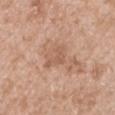Clinical impression: Imaged during a routine full-body skin examination; the lesion was not biopsied and no histopathology is available. Clinical summary: From the right upper arm. Cropped from a total-body skin-imaging series; the visible field is about 15 mm. Measured at roughly 3 mm in maximum diameter. An algorithmic analysis of the crop reported a mean CIELAB color near L≈57 a*≈21 b*≈31, a lesion–skin lightness drop of about 8, and a normalized lesion–skin contrast near 5. The software also gave a detector confidence of about 100 out of 100 that the crop contains a lesion. A male patient, in their 50s. Imaged with white-light lighting.